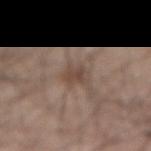The lesion-visualizer software estimated a lesion–skin lightness drop of about 8 and a lesion-to-skin contrast of about 6.5 (normalized; higher = more distinct). Captured under white-light illumination. A region of skin cropped from a whole-body photographic capture, roughly 15 mm wide. The lesion is located on the right forearm. A male subject in their mid- to late 30s. About 3 mm across.